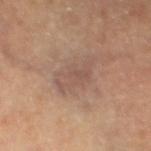Case summary:
- patient — male, approximately 60 years of age
- automated lesion analysis — roughly 6 lightness units darker than nearby skin and a lesion-to-skin contrast of about 5 (normalized; higher = more distinct); a nevus-likeness score of about 0/100 and lesion-presence confidence of about 100/100
- body site — the leg
- acquisition — 15 mm crop, total-body photography
- lesion diameter — about 5 mm